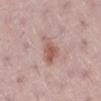Q: Is there a histopathology result?
A: catalogued during a skin exam; not biopsied
Q: Lesion location?
A: the right lower leg
Q: Who is the patient?
A: female, aged 48–52
Q: What is the imaging modality?
A: 15 mm crop, total-body photography